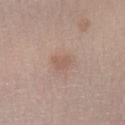This lesion was catalogued during total-body skin photography and was not selected for biopsy.
A 15 mm crop from a total-body photograph taken for skin-cancer surveillance.
The patient is a male aged around 35.
An algorithmic analysis of the crop reported a classifier nevus-likeness of about 5/100 and a lesion-detection confidence of about 100/100.
This is a white-light tile.
The lesion's longest dimension is about 2.5 mm.
On the leg.